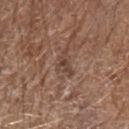Impression: Imaged during a routine full-body skin examination; the lesion was not biopsied and no histopathology is available. Background: Captured under white-light illumination. The lesion is on the left forearm. A male subject, aged 68 to 72. Cropped from a total-body skin-imaging series; the visible field is about 15 mm.